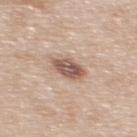Q: Was this lesion biopsied?
A: total-body-photography surveillance lesion; no biopsy
Q: Where on the body is the lesion?
A: the upper back
Q: What kind of image is this?
A: total-body-photography crop, ~15 mm field of view
Q: What lighting was used for the tile?
A: white-light illumination
Q: Patient demographics?
A: male, roughly 60 years of age